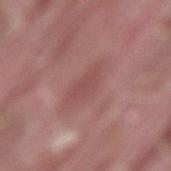Assessment:
This lesion was catalogued during total-body skin photography and was not selected for biopsy.
Context:
A male patient aged 38 to 42. This image is a 15 mm lesion crop taken from a total-body photograph. Located on the back. Captured under white-light illumination.